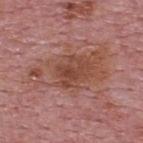{"biopsy_status": "not biopsied; imaged during a skin examination", "site": "upper back", "patient": {"sex": "male", "age_approx": 75}, "image": {"source": "total-body photography crop", "field_of_view_mm": 15}}